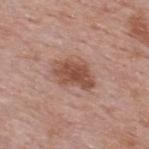* diameter — about 4.5 mm
* acquisition — ~15 mm crop, total-body skin-cancer survey
* patient — male, in their mid-50s
* illumination — white-light
* anatomic site — the upper back